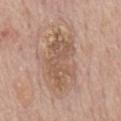workup: total-body-photography surveillance lesion; no biopsy
image-analysis metrics: a lesion-detection confidence of about 100/100
image source: ~15 mm crop, total-body skin-cancer survey
size: ≈7.5 mm
patient: male, approximately 75 years of age
site: the mid back